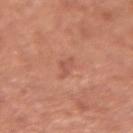follow-up: catalogued during a skin exam; not biopsied
lighting: white-light
image-analysis metrics: two-axis asymmetry of about 0.5; a lesion color around L≈55 a*≈26 b*≈30 in CIELAB and about 7 CIELAB-L* units darker than the surrounding skin; a border-irregularity rating of about 5.5/10, a color-variation rating of about 0/10, and a peripheral color-asymmetry measure near 0
lesion size: ≈3 mm
patient: female, aged 48 to 52
body site: the left forearm
imaging modality: ~15 mm crop, total-body skin-cancer survey